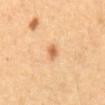No biopsy was performed on this lesion — it was imaged during a full skin examination and was not determined to be concerning. The lesion is located on the abdomen. Approximately 2.5 mm at its widest. Imaged with cross-polarized lighting. A male patient aged around 60. A region of skin cropped from a whole-body photographic capture, roughly 15 mm wide.